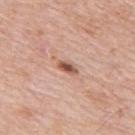Assessment:
No biopsy was performed on this lesion — it was imaged during a full skin examination and was not determined to be concerning.
Context:
A male subject aged 78 to 82. A 15 mm crop from a total-body photograph taken for skin-cancer surveillance. On the upper back. The total-body-photography lesion software estimated a lesion color around L≈55 a*≈23 b*≈29 in CIELAB and a normalized border contrast of about 9.5. Captured under white-light illumination. About 2.5 mm across.